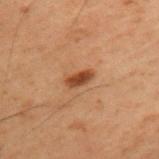No biopsy was performed on this lesion — it was imaged during a full skin examination and was not determined to be concerning. Imaged with cross-polarized lighting. The lesion's longest dimension is about 3 mm. A region of skin cropped from a whole-body photographic capture, roughly 15 mm wide. Automated image analysis of the tile measured a lesion-to-skin contrast of about 10 (normalized; higher = more distinct). The lesion is located on the back. A male patient, aged around 60.